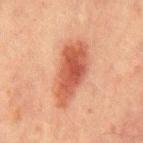Q: Was a biopsy performed?
A: no biopsy performed (imaged during a skin exam)
Q: How was this image acquired?
A: ~15 mm tile from a whole-body skin photo
Q: How was the tile lit?
A: cross-polarized
Q: Patient demographics?
A: male, aged approximately 65
Q: Where on the body is the lesion?
A: the chest
Q: Lesion size?
A: ≈7.5 mm